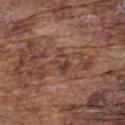The lesion is on the upper back. The lesion-visualizer software estimated an area of roughly 4.5 mm² and a shape-asymmetry score of about 0.35 (0 = symmetric). And it measured an automated nevus-likeness rating near 0 out of 100. About 2.5 mm across. A male patient in their mid- to late 70s. A close-up tile cropped from a whole-body skin photograph, about 15 mm across.